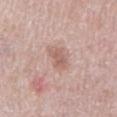Captured during whole-body skin photography for melanoma surveillance; the lesion was not biopsied.